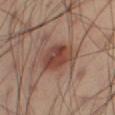This lesion was catalogued during total-body skin photography and was not selected for biopsy. About 4.5 mm across. A male subject aged approximately 40. From the right thigh. The tile uses cross-polarized illumination. A 15 mm crop from a total-body photograph taken for skin-cancer surveillance.